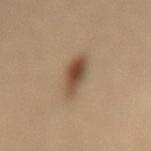Case summary:
- image — 15 mm crop, total-body photography
- subject — female, aged approximately 65
- automated lesion analysis — an area of roughly 7.5 mm², an eccentricity of roughly 0.75, and two-axis asymmetry of about 0.2; an average lesion color of about L≈47 a*≈16 b*≈30 (CIELAB), a lesion–skin lightness drop of about 13, and a normalized lesion–skin contrast near 9.5; an automated nevus-likeness rating near 100 out of 100 and lesion-presence confidence of about 100/100
- site — the back
- illumination — cross-polarized